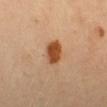biopsy status=catalogued during a skin exam; not biopsied | tile lighting=cross-polarized | image source=~15 mm crop, total-body skin-cancer survey | automated lesion analysis=an area of roughly 6.5 mm² and two-axis asymmetry of about 0.2; a nevus-likeness score of about 100/100 and lesion-presence confidence of about 100/100 | lesion size=~3 mm (longest diameter) | site=the head or neck | subject=female, aged 33–37.